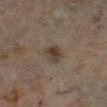Clinical summary:
This is a cross-polarized tile. A 15 mm close-up tile from a total-body photography series done for melanoma screening. Longest diameter approximately 3 mm. From the right lower leg. The patient is a female aged around 60.